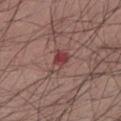Impression:
The lesion was photographed on a routine skin check and not biopsied; there is no pathology result.
Acquisition and patient details:
Captured under white-light illumination. A male subject, aged 38–42. Longest diameter approximately 3.5 mm. On the left lower leg. A 15 mm crop from a total-body photograph taken for skin-cancer surveillance.